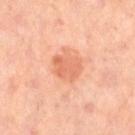The lesion was tiled from a total-body skin photograph and was not biopsied.
Approximately 3 mm at its widest.
Cropped from a whole-body photographic skin survey; the tile spans about 15 mm.
Imaged with cross-polarized lighting.
The lesion-visualizer software estimated a lesion area of about 7 mm², an outline eccentricity of about 0.5 (0 = round, 1 = elongated), and a shape-asymmetry score of about 0.25 (0 = symmetric).
The lesion is located on the lower back.
A female patient aged around 50.